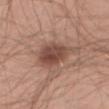follow-up = imaged on a skin check; not biopsied | patient = male, roughly 40 years of age | body site = the right thigh | lighting = white-light | diameter = ~5.5 mm (longest diameter) | imaging modality = ~15 mm crop, total-body skin-cancer survey.